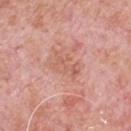No biopsy was performed on this lesion — it was imaged during a full skin examination and was not determined to be concerning.
The tile uses white-light illumination.
A lesion tile, about 15 mm wide, cut from a 3D total-body photograph.
Longest diameter approximately 3.5 mm.
Automated image analysis of the tile measured an average lesion color of about L≈60 a*≈25 b*≈30 (CIELAB), a lesion–skin lightness drop of about 6, and a normalized lesion–skin contrast near 5.
The lesion is on the chest.
A male subject aged around 80.